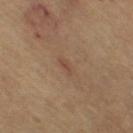{"biopsy_status": "not biopsied; imaged during a skin examination", "image": {"source": "total-body photography crop", "field_of_view_mm": 15}, "lighting": "cross-polarized", "patient": {"sex": "female", "age_approx": 65}, "site": "leg", "lesion_size": {"long_diameter_mm_approx": 2.5}}